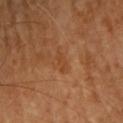Clinical impression: This lesion was catalogued during total-body skin photography and was not selected for biopsy. Acquisition and patient details: From the head or neck. A lesion tile, about 15 mm wide, cut from a 3D total-body photograph. Approximately 2.5 mm at its widest. Imaged with cross-polarized lighting. A female patient, aged approximately 60.